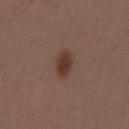Impression:
This lesion was catalogued during total-body skin photography and was not selected for biopsy.
Background:
The lesion-visualizer software estimated an area of roughly 5.5 mm², an outline eccentricity of about 0.85 (0 = round, 1 = elongated), and two-axis asymmetry of about 0.1. The software also gave a color-variation rating of about 2.5/10 and radial color variation of about 0.5. It also reported a nevus-likeness score of about 100/100 and a lesion-detection confidence of about 100/100. The patient is a male aged approximately 30. The tile uses white-light illumination. Measured at roughly 3.5 mm in maximum diameter. A 15 mm close-up extracted from a 3D total-body photography capture. Located on the back.